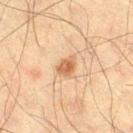Findings:
– illumination: cross-polarized
– imaging modality: total-body-photography crop, ~15 mm field of view
– subject: male, aged approximately 50
– location: the right thigh
– size: ~2.5 mm (longest diameter)
– image-analysis metrics: an eccentricity of roughly 0.6; a lesion color around L≈51 a*≈19 b*≈32 in CIELAB and a lesion-to-skin contrast of about 8 (normalized; higher = more distinct); a border-irregularity index near 3/10, a color-variation rating of about 3/10, and radial color variation of about 1; a nevus-likeness score of about 95/100